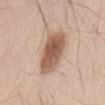follow-up = total-body-photography surveillance lesion; no biopsy | TBP lesion metrics = a border-irregularity rating of about 2/10 and a within-lesion color-variation index near 4.5/10; an automated nevus-likeness rating near 100 out of 100 and lesion-presence confidence of about 100/100 | body site = the abdomen | lighting = white-light | patient = male, aged around 45 | image = ~15 mm tile from a whole-body skin photo | diameter = about 6.5 mm.